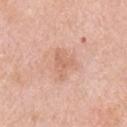Impression:
The lesion was tiled from a total-body skin photograph and was not biopsied.
Background:
A male subject about 80 years old. Captured under white-light illumination. A close-up tile cropped from a whole-body skin photograph, about 15 mm across. Longest diameter approximately 4 mm. On the right upper arm.